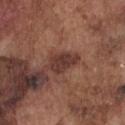This lesion was catalogued during total-body skin photography and was not selected for biopsy.
Longest diameter approximately 4 mm.
A 15 mm close-up tile from a total-body photography series done for melanoma screening.
The patient is a male in their mid-70s.
The lesion is on the front of the torso.
The lesion-visualizer software estimated a border-irregularity rating of about 3/10.